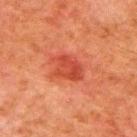Case summary:
– patient: male, approximately 80 years of age
– location: the upper back
– lighting: cross-polarized illumination
– acquisition: ~15 mm crop, total-body skin-cancer survey